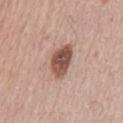biopsy_status: not biopsied; imaged during a skin examination
image:
  source: total-body photography crop
  field_of_view_mm: 15
site: front of the torso
automated_metrics:
  cielab_L: 52
  cielab_a: 20
  cielab_b: 26
  vs_skin_darker_L: 16.0
  vs_skin_contrast_norm: 10.5
lesion_size:
  long_diameter_mm_approx: 4.5
patient:
  sex: male
  age_approx: 65